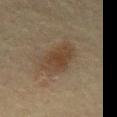Imaged during a routine full-body skin examination; the lesion was not biopsied and no histopathology is available. The lesion is on the abdomen. The subject is a male aged around 70. Cropped from a total-body skin-imaging series; the visible field is about 15 mm.